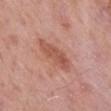Recorded during total-body skin imaging; not selected for excision or biopsy.